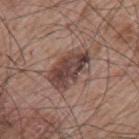Recorded during total-body skin imaging; not selected for excision or biopsy. A male patient aged 63–67. The tile uses white-light illumination. On the upper back. The lesion's longest dimension is about 6 mm. A close-up tile cropped from a whole-body skin photograph, about 15 mm across.